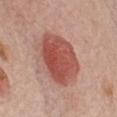Findings:
- imaging modality: ~15 mm tile from a whole-body skin photo
- illumination: white-light
- size: ≈7 mm
- site: the chest
- subject: female, in their 50s